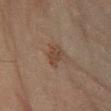Approximately 3 mm at its widest. The lesion is located on the left forearm. Cropped from a total-body skin-imaging series; the visible field is about 15 mm. A male patient aged 43–47.